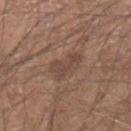follow-up: no biopsy performed (imaged during a skin exam)
patient: male, aged 48 to 52
tile lighting: white-light
acquisition: total-body-photography crop, ~15 mm field of view
diameter: about 3.5 mm
site: the left forearm